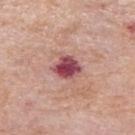Captured during whole-body skin photography for melanoma surveillance; the lesion was not biopsied. A female patient about 70 years old. Located on the arm. A close-up tile cropped from a whole-body skin photograph, about 15 mm across.